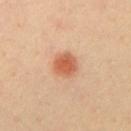| field | value |
|---|---|
| biopsy status | total-body-photography surveillance lesion; no biopsy |
| patient | male, roughly 40 years of age |
| site | the front of the torso |
| tile lighting | cross-polarized illumination |
| automated lesion analysis | a mean CIELAB color near L≈60 a*≈27 b*≈37, about 12 CIELAB-L* units darker than the surrounding skin, and a lesion-to-skin contrast of about 8 (normalized; higher = more distinct); a border-irregularity rating of about 1.5/10 and peripheral color asymmetry of about 1 |
| lesion diameter | ≈3 mm |
| acquisition | ~15 mm crop, total-body skin-cancer survey |